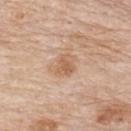workup: catalogued during a skin exam; not biopsied | imaging modality: ~15 mm crop, total-body skin-cancer survey | subject: male, approximately 85 years of age or older | TBP lesion metrics: an average lesion color of about L≈61 a*≈20 b*≈33 (CIELAB), roughly 9 lightness units darker than nearby skin, and a normalized border contrast of about 6.5; a border-irregularity rating of about 3/10, a within-lesion color-variation index near 2.5/10, and a peripheral color-asymmetry measure near 1; an automated nevus-likeness rating near 15 out of 100 | diameter: ≈3 mm | location: the upper back.